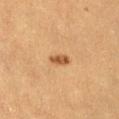<case>
<biopsy_status>not biopsied; imaged during a skin examination</biopsy_status>
<lesion_size>
  <long_diameter_mm_approx>2.5</long_diameter_mm_approx>
</lesion_size>
<image>
  <source>total-body photography crop</source>
  <field_of_view_mm>15</field_of_view_mm>
</image>
<lighting>cross-polarized</lighting>
<automated_metrics>
  <eccentricity>0.9</eccentricity>
  <shape_asymmetry>0.3</shape_asymmetry>
  <vs_skin_darker_L>13.0</vs_skin_darker_L>
  <vs_skin_contrast_norm>9.0</vs_skin_contrast_norm>
</automated_metrics>
<site>abdomen</site>
<patient>
  <sex>female</sex>
  <age_approx>30</age_approx>
</patient>
</case>